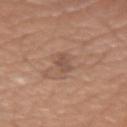Clinical impression:
No biopsy was performed on this lesion — it was imaged during a full skin examination and was not determined to be concerning.
Clinical summary:
Captured under white-light illumination. The lesion is located on the left upper arm. About 2.5 mm across. A male subject aged approximately 65. A 15 mm close-up extracted from a 3D total-body photography capture.